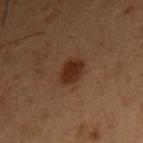Case summary:
– workup · imaged on a skin check; not biopsied
– automated lesion analysis · a footprint of about 6 mm², an outline eccentricity of about 0.6 (0 = round, 1 = elongated), and a shape-asymmetry score of about 0.2 (0 = symmetric); a classifier nevus-likeness of about 95/100
– anatomic site · the right upper arm
– diameter · about 3 mm
– tile lighting · cross-polarized illumination
– image source · total-body-photography crop, ~15 mm field of view
– subject · male, in their mid-50s